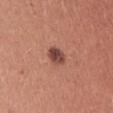This lesion was catalogued during total-body skin photography and was not selected for biopsy. The recorded lesion diameter is about 2.5 mm. A 15 mm close-up tile from a total-body photography series done for melanoma screening. From the abdomen. The lesion-visualizer software estimated a shape-asymmetry score of about 0.2 (0 = symmetric). A male patient aged 43–47.